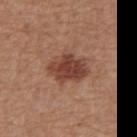workup = no biopsy performed (imaged during a skin exam)
imaging modality = total-body-photography crop, ~15 mm field of view
illumination = white-light illumination
automated lesion analysis = a lesion area of about 13 mm² and an eccentricity of roughly 0.6; about 12 CIELAB-L* units darker than the surrounding skin and a normalized border contrast of about 9; border irregularity of about 2.5 on a 0–10 scale, a color-variation rating of about 4/10, and peripheral color asymmetry of about 1; a nevus-likeness score of about 85/100 and a lesion-detection confidence of about 100/100
patient = male, aged around 65
anatomic site = the abdomen